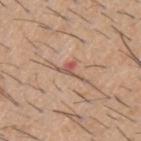follow-up: imaged on a skin check; not biopsied
image: ~15 mm crop, total-body skin-cancer survey
subject: male, aged 58–62
site: the upper back
lighting: white-light illumination